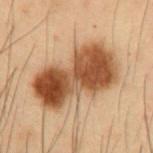Automated tile analysis of the lesion measured a border-irregularity index near 3.5/10, a within-lesion color-variation index near 7.5/10, and radial color variation of about 3.
Measured at roughly 9.5 mm in maximum diameter.
The lesion is located on the abdomen.
A roughly 15 mm field-of-view crop from a total-body skin photograph.
A male patient, aged 53–57.